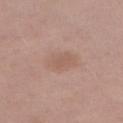The lesion was photographed on a routine skin check and not biopsied; there is no pathology result. A 15 mm crop from a total-body photograph taken for skin-cancer surveillance. Approximately 4 mm at its widest. The subject is a female approximately 40 years of age. From the right upper arm. This is a white-light tile. The lesion-visualizer software estimated a footprint of about 8.5 mm², a shape eccentricity near 0.65, and two-axis asymmetry of about 0.15. The software also gave a lesion–skin lightness drop of about 6 and a lesion-to-skin contrast of about 4.5 (normalized; higher = more distinct). The software also gave an automated nevus-likeness rating near 0 out of 100 and lesion-presence confidence of about 100/100.